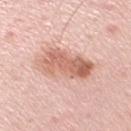Notes:
* illumination · white-light illumination
* acquisition · ~15 mm crop, total-body skin-cancer survey
* patient · female, roughly 40 years of age
* site · the right upper arm